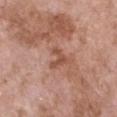| feature | finding |
|---|---|
| follow-up | total-body-photography surveillance lesion; no biopsy |
| subject | female, aged 73–77 |
| tile lighting | white-light illumination |
| image | ~15 mm tile from a whole-body skin photo |
| site | the chest |
| automated lesion analysis | a detector confidence of about 100 out of 100 that the crop contains a lesion |
| lesion diameter | ~2.5 mm (longest diameter) |